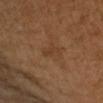Notes:
* workup — total-body-photography surveillance lesion; no biopsy
* tile lighting — cross-polarized
* size — ~3.5 mm (longest diameter)
* automated lesion analysis — a footprint of about 4 mm², an outline eccentricity of about 0.9 (0 = round, 1 = elongated), and a symmetry-axis asymmetry near 0.3; a normalized lesion–skin contrast near 4.5; a border-irregularity rating of about 3/10, a within-lesion color-variation index near 1/10, and radial color variation of about 0.5
* image source — total-body-photography crop, ~15 mm field of view
* subject — female, roughly 50 years of age
* site — the left upper arm